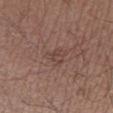Captured during whole-body skin photography for melanoma surveillance; the lesion was not biopsied. From the left lower leg. Longest diameter approximately 2.5 mm. This image is a 15 mm lesion crop taken from a total-body photograph. Automated tile analysis of the lesion measured a border-irregularity index near 4/10 and radial color variation of about 1. The analysis additionally found an automated nevus-likeness rating near 0 out of 100 and lesion-presence confidence of about 100/100. A male subject, approximately 60 years of age. Imaged with white-light lighting.